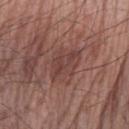{"biopsy_status": "not biopsied; imaged during a skin examination", "lighting": "white-light", "patient": {"sex": "male", "age_approx": 80}, "image": {"source": "total-body photography crop", "field_of_view_mm": 15}, "lesion_size": {"long_diameter_mm_approx": 4.5}, "site": "right forearm"}